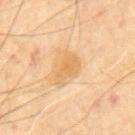A male subject aged 68–72. The lesion is located on the back. A 15 mm crop from a total-body photograph taken for skin-cancer surveillance.